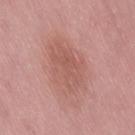Case summary:
– biopsy status — no biopsy performed (imaged during a skin exam)
– imaging modality — ~15 mm tile from a whole-body skin photo
– anatomic site — the right thigh
– patient — female, approximately 50 years of age